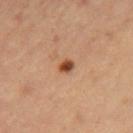notes: imaged on a skin check; not biopsied | subject: female, roughly 35 years of age | lesion diameter: ~2 mm (longest diameter) | site: the right thigh | illumination: cross-polarized | acquisition: 15 mm crop, total-body photography.